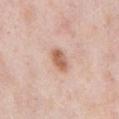Q: Is there a histopathology result?
A: total-body-photography surveillance lesion; no biopsy
Q: What is the imaging modality?
A: ~15 mm tile from a whole-body skin photo
Q: Lesion location?
A: the abdomen
Q: How large is the lesion?
A: ≈3.5 mm
Q: Illumination type?
A: white-light illumination
Q: What are the patient's age and sex?
A: male, aged 53 to 57